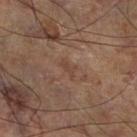follow-up: catalogued during a skin exam; not biopsied, lighting: cross-polarized illumination, location: the leg, imaging modality: ~15 mm tile from a whole-body skin photo, diameter: ~2.5 mm (longest diameter).